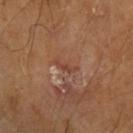Q: What lighting was used for the tile?
A: cross-polarized illumination
Q: What kind of image is this?
A: ~15 mm tile from a whole-body skin photo
Q: What did automated image analysis measure?
A: a lesion area of about 2.5 mm² and an outline eccentricity of about 0.95 (0 = round, 1 = elongated); a border-irregularity rating of about 6/10, a within-lesion color-variation index near 0/10, and peripheral color asymmetry of about 0; an automated nevus-likeness rating near 0 out of 100 and a detector confidence of about 60 out of 100 that the crop contains a lesion
Q: Where on the body is the lesion?
A: the left upper arm